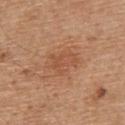Q: Is there a histopathology result?
A: total-body-photography surveillance lesion; no biopsy
Q: Patient demographics?
A: male, about 70 years old
Q: Where on the body is the lesion?
A: the upper back
Q: What kind of image is this?
A: 15 mm crop, total-body photography